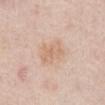Recorded during total-body skin imaging; not selected for excision or biopsy.
Automated image analysis of the tile measured about 7 CIELAB-L* units darker than the surrounding skin.
Captured under white-light illumination.
A male patient aged 73–77.
A 15 mm close-up extracted from a 3D total-body photography capture.
Approximately 4 mm at its widest.
The lesion is located on the abdomen.